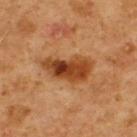The lesion was tiled from a total-body skin photograph and was not biopsied.
Captured under cross-polarized illumination.
The lesion's longest dimension is about 6 mm.
A 15 mm close-up tile from a total-body photography series done for melanoma screening.
Located on the upper back.
A male subject approximately 60 years of age.